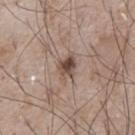The patient is a male aged approximately 75.
Longest diameter approximately 3 mm.
The total-body-photography lesion software estimated a lesion color around L≈48 a*≈16 b*≈24 in CIELAB and about 12 CIELAB-L* units darker than the surrounding skin.
This is a white-light tile.
A 15 mm crop from a total-body photograph taken for skin-cancer surveillance.
From the front of the torso.